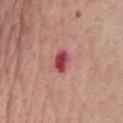Clinical summary:
This is a white-light tile. The recorded lesion diameter is about 3 mm. A region of skin cropped from a whole-body photographic capture, roughly 15 mm wide. The lesion is on the chest. A male patient, about 80 years old.